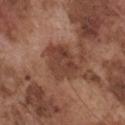notes: catalogued during a skin exam; not biopsied
subject: male, aged 73 to 77
site: the chest
acquisition: ~15 mm tile from a whole-body skin photo
size: ≈5 mm
tile lighting: white-light